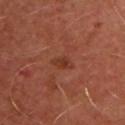Captured during whole-body skin photography for melanoma surveillance; the lesion was not biopsied.
The patient is a male aged around 50.
Automated image analysis of the tile measured an area of roughly 3 mm², an eccentricity of roughly 0.8, and two-axis asymmetry of about 0.35. And it measured a mean CIELAB color near L≈36 a*≈26 b*≈30 and a normalized border contrast of about 6. The analysis additionally found a border-irregularity rating of about 3/10, a within-lesion color-variation index near 2.5/10, and a peripheral color-asymmetry measure near 1.
Imaged with cross-polarized lighting.
Cropped from a total-body skin-imaging series; the visible field is about 15 mm.
The lesion is on the front of the torso.
The recorded lesion diameter is about 2.5 mm.